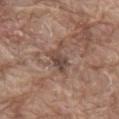Case summary:
* workup — imaged on a skin check; not biopsied
* tile lighting — white-light
* subject — male, in their 80s
* automated lesion analysis — an automated nevus-likeness rating near 0 out of 100 and a detector confidence of about 95 out of 100 that the crop contains a lesion
* anatomic site — the back
* size — ≈4 mm
* imaging modality — ~15 mm tile from a whole-body skin photo